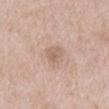The lesion was tiled from a total-body skin photograph and was not biopsied. Cropped from a total-body skin-imaging series; the visible field is about 15 mm. On the mid back. The lesion-visualizer software estimated a footprint of about 4.5 mm² and two-axis asymmetry of about 0.25. And it measured a border-irregularity rating of about 2.5/10, internal color variation of about 2.5 on a 0–10 scale, and a peripheral color-asymmetry measure near 1. The lesion's longest dimension is about 3 mm. Captured under white-light illumination. A male patient, in their mid- to late 60s.